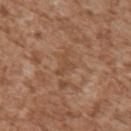No biopsy was performed on this lesion — it was imaged during a full skin examination and was not determined to be concerning. An algorithmic analysis of the crop reported a lesion color around L≈47 a*≈19 b*≈32 in CIELAB, a lesion–skin lightness drop of about 6, and a normalized border contrast of about 5. And it measured an automated nevus-likeness rating near 0 out of 100 and a lesion-detection confidence of about 95/100. Measured at roughly 3 mm in maximum diameter. Captured under white-light illumination. A male subject in their mid- to late 40s. A 15 mm crop from a total-body photograph taken for skin-cancer surveillance. From the right upper arm.